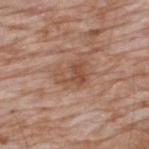• biopsy status · no biopsy performed (imaged during a skin exam)
• tile lighting · white-light
• location · the back
• acquisition · 15 mm crop, total-body photography
• subject · male, in their 60s